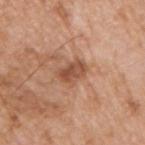biopsy status = catalogued during a skin exam; not biopsied | anatomic site = the right upper arm | patient = male, approximately 60 years of age | diameter = ~3 mm (longest diameter) | imaging modality = ~15 mm tile from a whole-body skin photo.